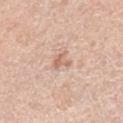Captured during whole-body skin photography for melanoma surveillance; the lesion was not biopsied. From the right lower leg. A roughly 15 mm field-of-view crop from a total-body skin photograph. The tile uses white-light illumination. Longest diameter approximately 2.5 mm. The subject is a female about 65 years old.